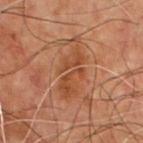biopsy status: catalogued during a skin exam; not biopsied | subject: male, about 70 years old | lesion size: about 6.5 mm | automated lesion analysis: internal color variation of about 2.5 on a 0–10 scale; an automated nevus-likeness rating near 0 out of 100 and a detector confidence of about 100 out of 100 that the crop contains a lesion | site: the front of the torso | illumination: cross-polarized illumination | acquisition: ~15 mm crop, total-body skin-cancer survey.